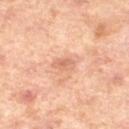| field | value |
|---|---|
| workup | catalogued during a skin exam; not biopsied |
| subject | female, in their 40s |
| TBP lesion metrics | a lesion color around L≈66 a*≈25 b*≈34 in CIELAB, roughly 9 lightness units darker than nearby skin, and a normalized border contrast of about 5.5 |
| body site | the left lower leg |
| tile lighting | cross-polarized illumination |
| image source | total-body-photography crop, ~15 mm field of view |